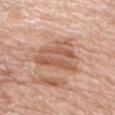Impression: Imaged during a routine full-body skin examination; the lesion was not biopsied and no histopathology is available.